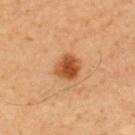Captured during whole-body skin photography for melanoma surveillance; the lesion was not biopsied.
A male patient, aged 48–52.
A close-up tile cropped from a whole-body skin photograph, about 15 mm across.
From the upper back.
An algorithmic analysis of the crop reported a border-irregularity rating of about 1.5/10, a within-lesion color-variation index near 5/10, and radial color variation of about 1.5.
Captured under cross-polarized illumination.
The recorded lesion diameter is about 3 mm.